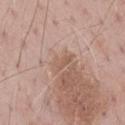Q: Was a biopsy performed?
A: no biopsy performed (imaged during a skin exam)
Q: What is the imaging modality?
A: ~15 mm tile from a whole-body skin photo
Q: What is the lesion's diameter?
A: ~3 mm (longest diameter)
Q: How was the tile lit?
A: white-light
Q: Where on the body is the lesion?
A: the mid back
Q: Who is the patient?
A: male, about 70 years old
Q: Automated lesion metrics?
A: a footprint of about 3 mm², a shape eccentricity near 0.9, and two-axis asymmetry of about 0.35; an average lesion color of about L≈57 a*≈19 b*≈28 (CIELAB) and roughly 8 lightness units darker than nearby skin; an automated nevus-likeness rating near 0 out of 100 and a detector confidence of about 100 out of 100 that the crop contains a lesion